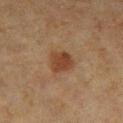Notes:
• biopsy status: total-body-photography surveillance lesion; no biopsy
• image-analysis metrics: a border-irregularity rating of about 2.5/10, a color-variation rating of about 2/10, and a peripheral color-asymmetry measure near 0.5; a classifier nevus-likeness of about 95/100 and a lesion-detection confidence of about 100/100
• location: the right lower leg
• illumination: cross-polarized
• subject: female, aged 53 to 57
• image: ~15 mm tile from a whole-body skin photo
• size: ~3 mm (longest diameter)